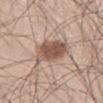  biopsy_status: not biopsied; imaged during a skin examination
  lesion_size:
    long_diameter_mm_approx: 5.0
  automated_metrics:
    area_mm2_approx: 12.0
    cielab_L: 53
    cielab_a: 17
    cielab_b: 27
    vs_skin_darker_L: 14.0
    nevus_likeness_0_100: 95
    lesion_detection_confidence_0_100: 100
  lighting: white-light
  patient:
    sex: male
    age_approx: 45
  image:
    source: total-body photography crop
    field_of_view_mm: 15
  site: left thigh